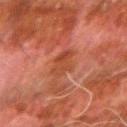Impression: Captured during whole-body skin photography for melanoma surveillance; the lesion was not biopsied. Background: The tile uses cross-polarized illumination. The lesion's longest dimension is about 3.5 mm. The lesion is located on the right forearm. A 15 mm crop from a total-body photograph taken for skin-cancer surveillance. An algorithmic analysis of the crop reported a lesion area of about 6.5 mm², a shape eccentricity near 0.7, and a shape-asymmetry score of about 0.25 (0 = symmetric). The software also gave a lesion–skin lightness drop of about 6. The software also gave internal color variation of about 3 on a 0–10 scale and radial color variation of about 1. The software also gave a classifier nevus-likeness of about 0/100 and lesion-presence confidence of about 100/100. The patient is a male roughly 80 years of age.